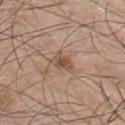This lesion was catalogued during total-body skin photography and was not selected for biopsy.
From the chest.
Cropped from a total-body skin-imaging series; the visible field is about 15 mm.
Automated tile analysis of the lesion measured a border-irregularity rating of about 3.5/10 and internal color variation of about 4.5 on a 0–10 scale.
Imaged with white-light lighting.
The recorded lesion diameter is about 3.5 mm.
The patient is a male aged approximately 45.